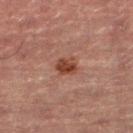{"biopsy_status": "not biopsied; imaged during a skin examination"}